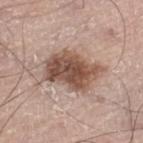Measured at roughly 6 mm in maximum diameter.
A male patient aged 68–72.
Cropped from a whole-body photographic skin survey; the tile spans about 15 mm.
Automated image analysis of the tile measured a nevus-likeness score of about 85/100 and a detector confidence of about 100 out of 100 that the crop contains a lesion.
On the left leg.